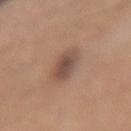imaging modality: ~15 mm crop, total-body skin-cancer survey
subject: female, about 45 years old
diameter: ~3.5 mm (longest diameter)
body site: the right forearm
automated metrics: a shape-asymmetry score of about 0.1 (0 = symmetric); a mean CIELAB color near L≈49 a*≈18 b*≈26, a lesion–skin lightness drop of about 10, and a lesion-to-skin contrast of about 7.5 (normalized; higher = more distinct); a border-irregularity index near 1.5/10, a within-lesion color-variation index near 4.5/10, and a peripheral color-asymmetry measure near 1.5; an automated nevus-likeness rating near 55 out of 100